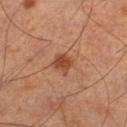Q: Was a biopsy performed?
A: catalogued during a skin exam; not biopsied
Q: What is the anatomic site?
A: the left lower leg
Q: What kind of image is this?
A: 15 mm crop, total-body photography
Q: Illumination type?
A: cross-polarized
Q: Patient demographics?
A: male, roughly 55 years of age
Q: Automated lesion metrics?
A: a shape eccentricity near 0.3 and a shape-asymmetry score of about 0.25 (0 = symmetric); a lesion-to-skin contrast of about 8 (normalized; higher = more distinct); border irregularity of about 2.5 on a 0–10 scale, internal color variation of about 2 on a 0–10 scale, and radial color variation of about 0.5
Q: How large is the lesion?
A: ≈2.5 mm